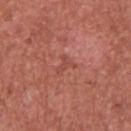automated metrics=an area of roughly 3 mm², a shape eccentricity near 0.7, and two-axis asymmetry of about 0.55; a detector confidence of about 100 out of 100 that the crop contains a lesion | imaging modality=~15 mm crop, total-body skin-cancer survey | subject=male, aged 43 to 47 | body site=the chest | diameter=≈2.5 mm | illumination=white-light.